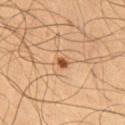A male patient about 65 years old. This is a cross-polarized tile. From the front of the torso. A roughly 15 mm field-of-view crop from a total-body skin photograph.